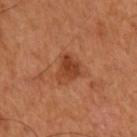The tile uses cross-polarized illumination.
On the right upper arm.
A roughly 15 mm field-of-view crop from a total-body skin photograph.
Measured at roughly 3.5 mm in maximum diameter.
A male subject, about 50 years old.